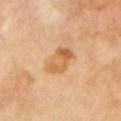<case>
<biopsy_status>not biopsied; imaged during a skin examination</biopsy_status>
<site>left upper arm</site>
<patient>
  <sex>male</sex>
  <age_approx>70</age_approx>
</patient>
<image>
  <source>total-body photography crop</source>
  <field_of_view_mm>15</field_of_view_mm>
</image>
<lighting>cross-polarized</lighting>
<lesion_size>
  <long_diameter_mm_approx>4.0</long_diameter_mm_approx>
</lesion_size>
</case>